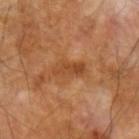Notes:
• biopsy status · total-body-photography surveillance lesion; no biopsy
• patient · male, aged 68 to 72
• imaging modality · ~15 mm tile from a whole-body skin photo
• site · the arm